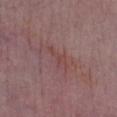Clinical impression: The lesion was tiled from a total-body skin photograph and was not biopsied. Acquisition and patient details: The lesion is on the left lower leg. The lesion's longest dimension is about 4 mm. The subject is a female roughly 60 years of age. Captured under white-light illumination. A roughly 15 mm field-of-view crop from a total-body skin photograph.